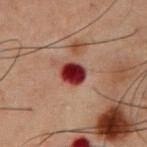  biopsy_status: not biopsied; imaged during a skin examination
  lesion_size:
    long_diameter_mm_approx: 3.0
  lighting: cross-polarized
  image:
    source: total-body photography crop
    field_of_view_mm: 15
  site: front of the torso
  patient:
    sex: male
    age_approx: 60